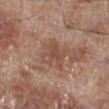workup: catalogued during a skin exam; not biopsied
subject: male, aged 68 to 72
imaging modality: ~15 mm crop, total-body skin-cancer survey
site: the right lower leg